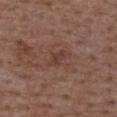{
  "biopsy_status": "not biopsied; imaged during a skin examination",
  "automated_metrics": {
    "cielab_L": 39,
    "cielab_a": 20,
    "cielab_b": 24,
    "vs_skin_darker_L": 7.0,
    "vs_skin_contrast_norm": 6.0,
    "border_irregularity_0_10": 3.0,
    "color_variation_0_10": 1.5,
    "peripheral_color_asymmetry": 0.5
  },
  "patient": {
    "sex": "male",
    "age_approx": 50
  },
  "lighting": "white-light",
  "site": "upper back",
  "image": {
    "source": "total-body photography crop",
    "field_of_view_mm": 15
  }
}